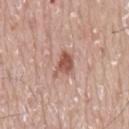Acquisition and patient details:
Automated tile analysis of the lesion measured roughly 12 lightness units darker than nearby skin and a normalized border contrast of about 8. And it measured a border-irregularity rating of about 4.5/10 and internal color variation of about 3 on a 0–10 scale. And it measured a nevus-likeness score of about 90/100 and a detector confidence of about 100 out of 100 that the crop contains a lesion. A male patient aged 73–77. The tile uses white-light illumination. A 15 mm close-up tile from a total-body photography series done for melanoma screening. Located on the mid back. Measured at roughly 3 mm in maximum diameter.